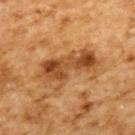Q: Was this lesion biopsied?
A: catalogued during a skin exam; not biopsied
Q: How was this image acquired?
A: ~15 mm crop, total-body skin-cancer survey
Q: Lesion location?
A: the upper back
Q: What lighting was used for the tile?
A: cross-polarized
Q: What are the patient's age and sex?
A: male, aged around 85 or older
Q: What is the lesion's diameter?
A: ~7 mm (longest diameter)
Q: What did automated image analysis measure?
A: an area of roughly 16 mm², an eccentricity of roughly 0.9, and a shape-asymmetry score of about 0.4 (0 = symmetric); a border-irregularity rating of about 6/10 and a within-lesion color-variation index near 6/10; a classifier nevus-likeness of about 5/100 and a detector confidence of about 100 out of 100 that the crop contains a lesion